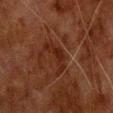The lesion was photographed on a routine skin check and not biopsied; there is no pathology result.